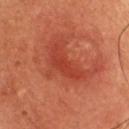This lesion was catalogued during total-body skin photography and was not selected for biopsy. A 15 mm close-up extracted from a 3D total-body photography capture. Longest diameter approximately 5 mm. The lesion is on the front of the torso. A male patient in their 60s. The lesion-visualizer software estimated a footprint of about 7 mm², an outline eccentricity of about 0.9 (0 = round, 1 = elongated), and two-axis asymmetry of about 0.55. It also reported border irregularity of about 6.5 on a 0–10 scale and radial color variation of about 0.5. Captured under cross-polarized illumination.